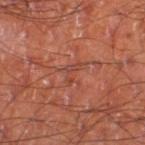{
  "biopsy_status": "not biopsied; imaged during a skin examination",
  "site": "leg",
  "lighting": "cross-polarized",
  "image": {
    "source": "total-body photography crop",
    "field_of_view_mm": 15
  },
  "automated_metrics": {
    "border_irregularity_0_10": 8.0,
    "nevus_likeness_0_100": 0,
    "lesion_detection_confidence_0_100": 50
  },
  "patient": {
    "sex": "male",
    "age_approx": 60
  }
}